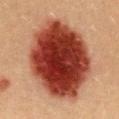The lesion was photographed on a routine skin check and not biopsied; there is no pathology result. A male subject aged 38–42. Longest diameter approximately 11 mm. A region of skin cropped from a whole-body photographic capture, roughly 15 mm wide. The lesion is located on the front of the torso. An algorithmic analysis of the crop reported a footprint of about 65 mm², an eccentricity of roughly 0.65, and a symmetry-axis asymmetry near 0.1. It also reported a lesion color around L≈33 a*≈27 b*≈27 in CIELAB and a normalized lesion–skin contrast near 16.